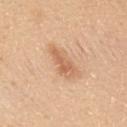Assessment: Captured during whole-body skin photography for melanoma surveillance; the lesion was not biopsied. Background: Measured at roughly 4.5 mm in maximum diameter. This is a white-light tile. Cropped from a total-body skin-imaging series; the visible field is about 15 mm. Automated image analysis of the tile measured an average lesion color of about L≈63 a*≈22 b*≈36 (CIELAB), roughly 10 lightness units darker than nearby skin, and a lesion-to-skin contrast of about 6.5 (normalized; higher = more distinct). And it measured a border-irregularity index near 4.5/10, a within-lesion color-variation index near 2/10, and peripheral color asymmetry of about 0.5. The analysis additionally found a nevus-likeness score of about 5/100 and a lesion-detection confidence of about 100/100. The patient is a male in their 30s. From the left upper arm.